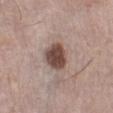Q: What are the patient's age and sex?
A: female, aged around 65
Q: What did automated image analysis measure?
A: a footprint of about 9 mm², an outline eccentricity of about 0.45 (0 = round, 1 = elongated), and two-axis asymmetry of about 0.15; a classifier nevus-likeness of about 90/100 and a lesion-detection confidence of about 100/100
Q: Where on the body is the lesion?
A: the left lower leg
Q: What is the imaging modality?
A: total-body-photography crop, ~15 mm field of view